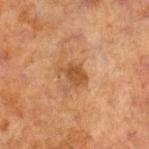Assessment: This lesion was catalogued during total-body skin photography and was not selected for biopsy. Context: From the leg. A region of skin cropped from a whole-body photographic capture, roughly 15 mm wide. Captured under cross-polarized illumination. A male subject, aged around 70.